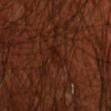This lesion was catalogued during total-body skin photography and was not selected for biopsy.
A close-up tile cropped from a whole-body skin photograph, about 15 mm across.
A male subject aged 68 to 72.
Longest diameter approximately 2.5 mm.
The tile uses cross-polarized illumination.
On the front of the torso.